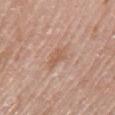The lesion was tiled from a total-body skin photograph and was not biopsied.
A region of skin cropped from a whole-body photographic capture, roughly 15 mm wide.
The lesion is located on the left upper arm.
A female patient about 65 years old.